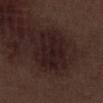Part of a total-body skin-imaging series; this lesion was reviewed on a skin check and was not flagged for biopsy.
The lesion-visualizer software estimated an area of roughly 21 mm², a shape eccentricity near 0.8, and a symmetry-axis asymmetry near 0.2. And it measured roughly 7 lightness units darker than nearby skin and a lesion-to-skin contrast of about 9.5 (normalized; higher = more distinct). The analysis additionally found a border-irregularity index near 2.5/10, a within-lesion color-variation index near 3/10, and radial color variation of about 1. It also reported a classifier nevus-likeness of about 10/100 and a lesion-detection confidence of about 95/100.
This is a white-light tile.
The lesion's longest dimension is about 6.5 mm.
The patient is a male about 70 years old.
Located on the right lower leg.
A 15 mm close-up extracted from a 3D total-body photography capture.